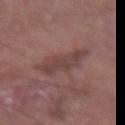No biopsy was performed on this lesion — it was imaged during a full skin examination and was not determined to be concerning. The lesion-visualizer software estimated a lesion area of about 11 mm², a shape eccentricity near 0.8, and a shape-asymmetry score of about 0.4 (0 = symmetric). The tile uses white-light illumination. A close-up tile cropped from a whole-body skin photograph, about 15 mm across. The lesion's longest dimension is about 5 mm. The lesion is located on the right forearm. A male subject, approximately 70 years of age.